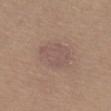biopsy status=imaged on a skin check; not biopsied | subject=male, in their mid-60s | image source=total-body-photography crop, ~15 mm field of view | anatomic site=the left thigh.